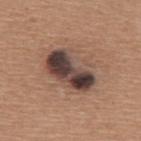Findings:
* workup · imaged on a skin check; not biopsied
* site · the back
* image · ~15 mm tile from a whole-body skin photo
* subject · female, approximately 55 years of age